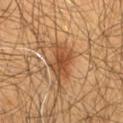Part of a total-body skin-imaging series; this lesion was reviewed on a skin check and was not flagged for biopsy. About 4 mm across. The lesion is located on the front of the torso. The tile uses cross-polarized illumination. Cropped from a total-body skin-imaging series; the visible field is about 15 mm. The patient is a male aged 58–62.